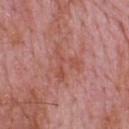Notes:
* follow-up: no biopsy performed (imaged during a skin exam)
* tile lighting: white-light
* imaging modality: 15 mm crop, total-body photography
* size: about 4.5 mm
* subject: male, in their mid-60s
* body site: the back